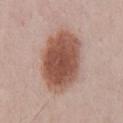{
  "biopsy_status": "not biopsied; imaged during a skin examination",
  "image": {
    "source": "total-body photography crop",
    "field_of_view_mm": 15
  },
  "lighting": "white-light",
  "lesion_size": {
    "long_diameter_mm_approx": 8.0
  },
  "site": "front of the torso",
  "patient": {
    "sex": "male",
    "age_approx": 35
  }
}